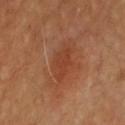biopsy status: total-body-photography surveillance lesion; no biopsy | patient: male, approximately 55 years of age | location: the chest | image source: 15 mm crop, total-body photography.